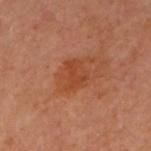Clinical impression:
Captured during whole-body skin photography for melanoma surveillance; the lesion was not biopsied.
Background:
From the left arm. The patient is a female aged around 60. The total-body-photography lesion software estimated an average lesion color of about L≈39 a*≈25 b*≈32 (CIELAB) and about 6 CIELAB-L* units darker than the surrounding skin. And it measured border irregularity of about 3.5 on a 0–10 scale, a within-lesion color-variation index near 2.5/10, and peripheral color asymmetry of about 1. Captured under cross-polarized illumination. About 3.5 mm across. A lesion tile, about 15 mm wide, cut from a 3D total-body photograph.